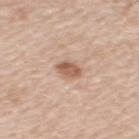workup — catalogued during a skin exam; not biopsied